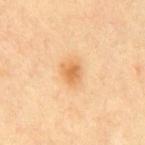workup: imaged on a skin check; not biopsied | body site: the abdomen | tile lighting: cross-polarized | image source: total-body-photography crop, ~15 mm field of view | patient: male, roughly 70 years of age | lesion size: about 3 mm | automated metrics: a lesion area of about 6 mm², a shape eccentricity near 0.6, and a shape-asymmetry score of about 0.25 (0 = symmetric); a nevus-likeness score of about 85/100 and a lesion-detection confidence of about 100/100.